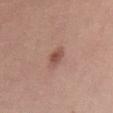follow-up — imaged on a skin check; not biopsied
automated metrics — a lesion area of about 3.5 mm²; roughly 11 lightness units darker than nearby skin
image — 15 mm crop, total-body photography
patient — male, in their 30s
tile lighting — white-light
lesion size — ~3 mm (longest diameter)